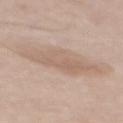Case summary:
- notes — total-body-photography surveillance lesion; no biopsy
- patient — male, aged around 65
- location — the mid back
- image source — ~15 mm tile from a whole-body skin photo
- image-analysis metrics — a lesion area of about 16 mm², a shape eccentricity near 0.9, and a shape-asymmetry score of about 0.35 (0 = symmetric); a lesion color around L≈62 a*≈15 b*≈27 in CIELAB, a lesion–skin lightness drop of about 7, and a normalized lesion–skin contrast near 5; a lesion-detection confidence of about 90/100
- diameter — ~7.5 mm (longest diameter)
- lighting — white-light illumination